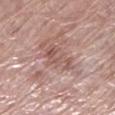Clinical impression: Captured during whole-body skin photography for melanoma surveillance; the lesion was not biopsied.